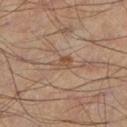Captured during whole-body skin photography for melanoma surveillance; the lesion was not biopsied. On the left lower leg. Automated image analysis of the tile measured a nevus-likeness score of about 0/100 and a lesion-detection confidence of about 100/100. A close-up tile cropped from a whole-body skin photograph, about 15 mm across. A male patient aged 53 to 57. This is a cross-polarized tile.